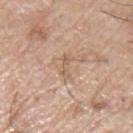{"biopsy_status": "not biopsied; imaged during a skin examination", "image": {"source": "total-body photography crop", "field_of_view_mm": 15}, "patient": {"sex": "male", "age_approx": 65}, "automated_metrics": {"eccentricity": 0.95, "shape_asymmetry": 0.35, "cielab_L": 60, "cielab_a": 16, "cielab_b": 30, "vs_skin_darker_L": 7.0, "border_irregularity_0_10": 4.5, "color_variation_0_10": 0.0, "peripheral_color_asymmetry": 0.0, "nevus_likeness_0_100": 0, "lesion_detection_confidence_0_100": 70}, "lesion_size": {"long_diameter_mm_approx": 3.5}, "lighting": "white-light", "site": "left upper arm"}